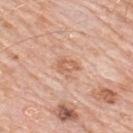Case summary:
- biopsy status: total-body-photography surveillance lesion; no biopsy
- acquisition: 15 mm crop, total-body photography
- lesion size: about 2.5 mm
- site: the upper back
- lighting: white-light
- TBP lesion metrics: a normalized lesion–skin contrast near 6; a border-irregularity index near 4/10, internal color variation of about 3.5 on a 0–10 scale, and peripheral color asymmetry of about 1.5; a lesion-detection confidence of about 100/100
- subject: male, approximately 80 years of age